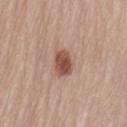Q: What is the imaging modality?
A: 15 mm crop, total-body photography
Q: Who is the patient?
A: female, in their mid- to late 70s
Q: Where on the body is the lesion?
A: the lower back